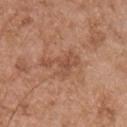workup: total-body-photography surveillance lesion; no biopsy
patient: male, aged 73 to 77
acquisition: ~15 mm tile from a whole-body skin photo
body site: the right upper arm
diameter: about 4.5 mm